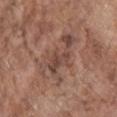Findings:
• workup — total-body-photography surveillance lesion; no biopsy
• patient — male, approximately 70 years of age
• imaging modality — 15 mm crop, total-body photography
• lighting — white-light illumination
• anatomic site — the chest
• size — ≈6 mm